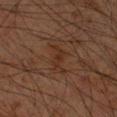Impression:
Imaged during a routine full-body skin examination; the lesion was not biopsied and no histopathology is available.
Clinical summary:
Automated image analysis of the tile measured an area of roughly 2.5 mm², an outline eccentricity of about 0.9 (0 = round, 1 = elongated), and a shape-asymmetry score of about 0.3 (0 = symmetric). The software also gave an average lesion color of about L≈23 a*≈16 b*≈23 (CIELAB), roughly 4 lightness units darker than nearby skin, and a lesion-to-skin contrast of about 5 (normalized; higher = more distinct). The analysis additionally found internal color variation of about 0 on a 0–10 scale and a peripheral color-asymmetry measure near 0. The software also gave a lesion-detection confidence of about 100/100. From the right upper arm. This image is a 15 mm lesion crop taken from a total-body photograph. A male subject, aged approximately 65. Imaged with cross-polarized lighting.